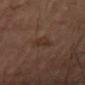Part of a total-body skin-imaging series; this lesion was reviewed on a skin check and was not flagged for biopsy.
Cropped from a whole-body photographic skin survey; the tile spans about 15 mm.
The tile uses cross-polarized illumination.
A male patient about 70 years old.
About 2.5 mm across.
From the right thigh.
The total-body-photography lesion software estimated an area of roughly 4.5 mm² and an eccentricity of roughly 0.6. The analysis additionally found an automated nevus-likeness rating near 0 out of 100 and a detector confidence of about 100 out of 100 that the crop contains a lesion.